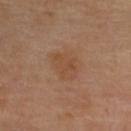The lesion was tiled from a total-body skin photograph and was not biopsied. The subject is a female roughly 40 years of age. The lesion is on the upper back. A roughly 15 mm field-of-view crop from a total-body skin photograph. The lesion-visualizer software estimated a footprint of about 8 mm², an eccentricity of roughly 0.55, and a shape-asymmetry score of about 0.25 (0 = symmetric). The analysis additionally found a lesion color around L≈48 a*≈19 b*≈33 in CIELAB, about 5 CIELAB-L* units darker than the surrounding skin, and a lesion-to-skin contrast of about 5 (normalized; higher = more distinct). And it measured border irregularity of about 3 on a 0–10 scale, a within-lesion color-variation index near 2/10, and radial color variation of about 1. The analysis additionally found a classifier nevus-likeness of about 0/100 and lesion-presence confidence of about 100/100. Imaged with cross-polarized lighting. The recorded lesion diameter is about 3.5 mm.